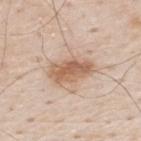No biopsy was performed on this lesion — it was imaged during a full skin examination and was not determined to be concerning.
A roughly 15 mm field-of-view crop from a total-body skin photograph.
The lesion-visualizer software estimated a classifier nevus-likeness of about 55/100 and a detector confidence of about 100 out of 100 that the crop contains a lesion.
A male subject in their 80s.
On the upper back.